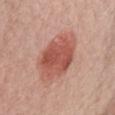{"biopsy_status": "not biopsied; imaged during a skin examination", "lesion_size": {"long_diameter_mm_approx": 7.5}, "automated_metrics": {"cielab_L": 55, "cielab_a": 27, "cielab_b": 28, "vs_skin_contrast_norm": 8.0, "color_variation_0_10": 4.5, "peripheral_color_asymmetry": 1.0, "nevus_likeness_0_100": 100}, "patient": {"sex": "female", "age_approx": 50}, "image": {"source": "total-body photography crop", "field_of_view_mm": 15}, "lighting": "white-light", "site": "chest"}